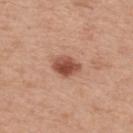notes: imaged on a skin check; not biopsied
subject: male, aged approximately 65
lesion size: ≈3.5 mm
body site: the upper back
lighting: white-light illumination
image source: total-body-photography crop, ~15 mm field of view
image-analysis metrics: an average lesion color of about L≈51 a*≈25 b*≈30 (CIELAB) and a normalized border contrast of about 10; a border-irregularity rating of about 2/10 and a color-variation rating of about 4.5/10; an automated nevus-likeness rating near 100 out of 100